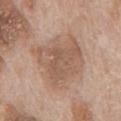Assessment:
This lesion was catalogued during total-body skin photography and was not selected for biopsy.
Image and clinical context:
The lesion is on the chest. Longest diameter approximately 6 mm. Captured under white-light illumination. A roughly 15 mm field-of-view crop from a total-body skin photograph. The patient is a male aged approximately 70.